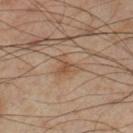follow-up — total-body-photography surveillance lesion; no biopsy
automated lesion analysis — an average lesion color of about L≈51 a*≈18 b*≈31 (CIELAB), roughly 7 lightness units darker than nearby skin, and a normalized border contrast of about 6; a border-irregularity index near 6/10, internal color variation of about 1.5 on a 0–10 scale, and peripheral color asymmetry of about 0.5; a nevus-likeness score of about 5/100 and lesion-presence confidence of about 100/100
lesion size — ≈3 mm
image — ~15 mm crop, total-body skin-cancer survey
patient — male, about 55 years old
site — the left lower leg